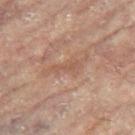| key | value |
|---|---|
| follow-up | total-body-photography surveillance lesion; no biopsy |
| site | the left leg |
| illumination | cross-polarized illumination |
| image | 15 mm crop, total-body photography |
| patient | female, in their 80s |